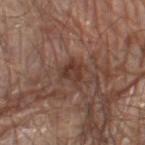The lesion was tiled from a total-body skin photograph and was not biopsied. The lesion is located on the left thigh. The tile uses white-light illumination. A male patient, roughly 70 years of age. A region of skin cropped from a whole-body photographic capture, roughly 15 mm wide. About 2.5 mm across. Automated image analysis of the tile measured a footprint of about 5 mm², a shape eccentricity near 0.4, and two-axis asymmetry of about 0.3. It also reported a normalized lesion–skin contrast near 7.5. And it measured a border-irregularity index near 3.5/10 and a within-lesion color-variation index near 3/10.